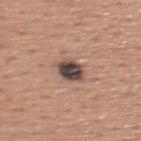follow-up: catalogued during a skin exam; not biopsied
image: 15 mm crop, total-body photography
subject: male, about 45 years old
anatomic site: the upper back